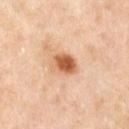Impression: Imaged during a routine full-body skin examination; the lesion was not biopsied and no histopathology is available. Acquisition and patient details: The lesion's longest dimension is about 3.5 mm. The lesion is on the leg. A female subject, aged approximately 50. This is a cross-polarized tile. A close-up tile cropped from a whole-body skin photograph, about 15 mm across.